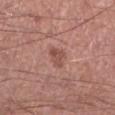workup = catalogued during a skin exam; not biopsied
site = the right lower leg
image source = 15 mm crop, total-body photography
subject = male, aged around 45